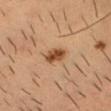Q: How was this image acquired?
A: ~15 mm tile from a whole-body skin photo
Q: What lighting was used for the tile?
A: cross-polarized
Q: Lesion size?
A: ~3 mm (longest diameter)
Q: Where on the body is the lesion?
A: the head or neck
Q: Who is the patient?
A: male, aged approximately 35